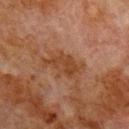Captured during whole-body skin photography for melanoma surveillance; the lesion was not biopsied.
A lesion tile, about 15 mm wide, cut from a 3D total-body photograph.
From the chest.
The subject is a male aged around 80.